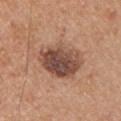This lesion was catalogued during total-body skin photography and was not selected for biopsy.
The patient is a male approximately 30 years of age.
An algorithmic analysis of the crop reported a lesion area of about 19 mm² and a symmetry-axis asymmetry near 0.2. And it measured about 14 CIELAB-L* units darker than the surrounding skin. It also reported border irregularity of about 2 on a 0–10 scale, a color-variation rating of about 7.5/10, and peripheral color asymmetry of about 2.5. And it measured an automated nevus-likeness rating near 5 out of 100.
Longest diameter approximately 5.5 mm.
Cropped from a total-body skin-imaging series; the visible field is about 15 mm.
Located on the arm.
Captured under white-light illumination.